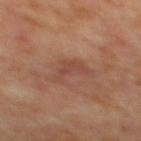Context:
Imaged with cross-polarized lighting. A patient approximately 65 years of age. The lesion is located on the mid back. About 4.5 mm across. A 15 mm close-up tile from a total-body photography series done for melanoma screening. The lesion-visualizer software estimated a nevus-likeness score of about 0/100 and a lesion-detection confidence of about 100/100.